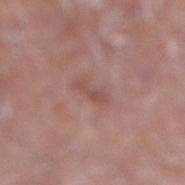Assessment: Recorded during total-body skin imaging; not selected for excision or biopsy. Image and clinical context: Captured under white-light illumination. A male patient aged approximately 70. A 15 mm close-up tile from a total-body photography series done for melanoma screening. The lesion is located on the right lower leg. Automated tile analysis of the lesion measured a footprint of about 2 mm², an eccentricity of roughly 0.95, and two-axis asymmetry of about 0.35. The software also gave an average lesion color of about L≈49 a*≈20 b*≈22 (CIELAB) and a normalized lesion–skin contrast near 5.5. Longest diameter approximately 2.5 mm.